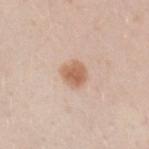Context:
A female patient, aged 18 to 22. On the left forearm. Measured at roughly 3 mm in maximum diameter. A roughly 15 mm field-of-view crop from a total-body skin photograph. This is a white-light tile.